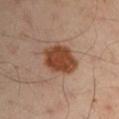Imaged during a routine full-body skin examination; the lesion was not biopsied and no histopathology is available.
The patient is a male about 50 years old.
Captured under cross-polarized illumination.
Cropped from a total-body skin-imaging series; the visible field is about 15 mm.
Located on the left upper arm.